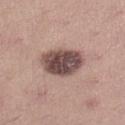{
  "biopsy_status": "not biopsied; imaged during a skin examination",
  "lesion_size": {
    "long_diameter_mm_approx": 5.0
  },
  "lighting": "white-light",
  "image": {
    "source": "total-body photography crop",
    "field_of_view_mm": 15
  },
  "site": "left lower leg",
  "patient": {
    "sex": "female",
    "age_approx": 25
  }
}